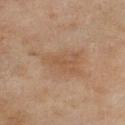Recorded during total-body skin imaging; not selected for excision or biopsy. A female patient approximately 60 years of age. A 15 mm crop from a total-body photograph taken for skin-cancer surveillance. The lesion is on the left lower leg.